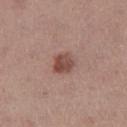<tbp_lesion>
  <biopsy_status>not biopsied; imaged during a skin examination</biopsy_status>
  <site>left thigh</site>
  <patient>
    <sex>female</sex>
    <age_approx>55</age_approx>
  </patient>
  <image>
    <source>total-body photography crop</source>
    <field_of_view_mm>15</field_of_view_mm>
  </image>
  <lighting>white-light</lighting>
  <lesion_size>
    <long_diameter_mm_approx>2.5</long_diameter_mm_approx>
  </lesion_size>
</tbp_lesion>